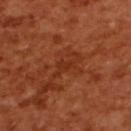notes — total-body-photography surveillance lesion; no biopsy
location — the upper back
patient — female, about 55 years old
image — 15 mm crop, total-body photography
lesion diameter — ~4 mm (longest diameter)
TBP lesion metrics — a footprint of about 4.5 mm², a shape eccentricity near 0.95, and two-axis asymmetry of about 0.6; a border-irregularity rating of about 7.5/10, internal color variation of about 1 on a 0–10 scale, and peripheral color asymmetry of about 0.5; a classifier nevus-likeness of about 0/100 and lesion-presence confidence of about 95/100